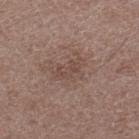{"site": "right lower leg", "image": {"source": "total-body photography crop", "field_of_view_mm": 15}, "lesion_size": {"long_diameter_mm_approx": 4.0}, "patient": {"sex": "male", "age_approx": 50}}